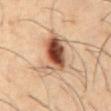Q: Was this lesion biopsied?
A: imaged on a skin check; not biopsied
Q: Lesion size?
A: ~6.5 mm (longest diameter)
Q: Where on the body is the lesion?
A: the abdomen
Q: What did automated image analysis measure?
A: an outline eccentricity of about 0.8 (0 = round, 1 = elongated) and a shape-asymmetry score of about 0.35 (0 = symmetric); border irregularity of about 4 on a 0–10 scale, internal color variation of about 10 on a 0–10 scale, and radial color variation of about 5; a classifier nevus-likeness of about 95/100 and a lesion-detection confidence of about 100/100
Q: What are the patient's age and sex?
A: male, in their mid- to late 50s
Q: What kind of image is this?
A: 15 mm crop, total-body photography